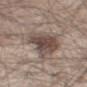Clinical impression: Imaged during a routine full-body skin examination; the lesion was not biopsied and no histopathology is available. Clinical summary: On the right thigh. A male subject in their mid- to late 40s. The lesion's longest dimension is about 6 mm. This image is a 15 mm lesion crop taken from a total-body photograph.